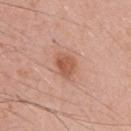site: the chest
patient: male, in their mid- to late 50s
illumination: white-light illumination
imaging modality: total-body-photography crop, ~15 mm field of view
automated metrics: a footprint of about 6 mm², an outline eccentricity of about 0.65 (0 = round, 1 = elongated), and a symmetry-axis asymmetry near 0.25; a border-irregularity rating of about 2/10 and a within-lesion color-variation index near 3/10
lesion diameter: ≈3 mm